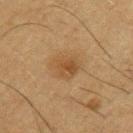Recorded during total-body skin imaging; not selected for excision or biopsy. About 3.5 mm across. The lesion is located on the left upper arm. A male patient roughly 35 years of age. A lesion tile, about 15 mm wide, cut from a 3D total-body photograph. Captured under cross-polarized illumination. The lesion-visualizer software estimated a lesion area of about 6.5 mm², an eccentricity of roughly 0.5, and a shape-asymmetry score of about 0.2 (0 = symmetric). And it measured a color-variation rating of about 3/10 and radial color variation of about 1. And it measured an automated nevus-likeness rating near 50 out of 100 and lesion-presence confidence of about 100/100.